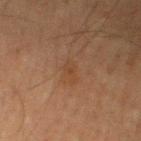{
  "biopsy_status": "not biopsied; imaged during a skin examination",
  "lighting": "cross-polarized",
  "site": "left lower leg",
  "image": {
    "source": "total-body photography crop",
    "field_of_view_mm": 15
  },
  "patient": {
    "sex": "male",
    "age_approx": 50
  }
}